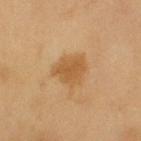– follow-up: total-body-photography surveillance lesion; no biopsy
– location: the left upper arm
– image source: ~15 mm crop, total-body skin-cancer survey
– patient: male, aged 58 to 62